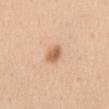notes — total-body-photography surveillance lesion; no biopsy | tile lighting — white-light illumination | anatomic site — the abdomen | lesion diameter — about 3 mm | patient — female, aged 43 to 47 | image source — ~15 mm crop, total-body skin-cancer survey | TBP lesion metrics — a lesion area of about 4 mm², a shape eccentricity near 0.75, and a shape-asymmetry score of about 0.3 (0 = symmetric); roughly 12 lightness units darker than nearby skin; a classifier nevus-likeness of about 90/100 and a detector confidence of about 100 out of 100 that the crop contains a lesion.